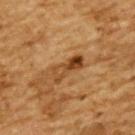notes — no biopsy performed (imaged during a skin exam) | subject — male, roughly 85 years of age or older | lesion diameter — ~4 mm (longest diameter) | acquisition — 15 mm crop, total-body photography | lighting — cross-polarized illumination | anatomic site — the upper back | automated lesion analysis — an area of roughly 6 mm², an eccentricity of roughly 0.9, and a shape-asymmetry score of about 0.25 (0 = symmetric); a lesion–skin lightness drop of about 10 and a normalized border contrast of about 8.5; a within-lesion color-variation index near 9.5/10 and radial color variation of about 3.5; a classifier nevus-likeness of about 10/100 and lesion-presence confidence of about 100/100.